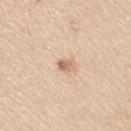tile lighting = white-light illumination
image source = ~15 mm crop, total-body skin-cancer survey
site = the right upper arm
size = ≈2 mm
subject = female, aged 23–27